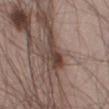Case summary:
- notes · total-body-photography surveillance lesion; no biopsy
- anatomic site · the left thigh
- imaging modality · total-body-photography crop, ~15 mm field of view
- patient · male, about 55 years old